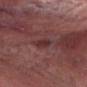Case summary:
- biopsy status: catalogued during a skin exam; not biopsied
- size: ≈3 mm
- lighting: white-light illumination
- location: the head or neck
- image source: ~15 mm tile from a whole-body skin photo
- patient: male, aged approximately 75